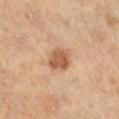biopsy status: total-body-photography surveillance lesion; no biopsy
patient: female, in their mid- to late 50s
illumination: cross-polarized
image: ~15 mm tile from a whole-body skin photo
diameter: about 3 mm
automated lesion analysis: an eccentricity of roughly 0.5 and a shape-asymmetry score of about 0.15 (0 = symmetric); an average lesion color of about L≈57 a*≈22 b*≈35 (CIELAB), roughly 12 lightness units darker than nearby skin, and a lesion-to-skin contrast of about 8 (normalized; higher = more distinct); border irregularity of about 1.5 on a 0–10 scale and a color-variation rating of about 2.5/10
site: the right lower leg